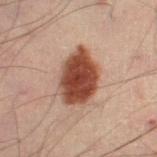Findings:
* follow-up — imaged on a skin check; not biopsied
* patient — male, aged 48–52
* image-analysis metrics — a lesion area of about 18 mm², an outline eccentricity of about 0.75 (0 = round, 1 = elongated), and a shape-asymmetry score of about 0.15 (0 = symmetric); a color-variation rating of about 4/10 and radial color variation of about 1
* lighting — cross-polarized illumination
* diameter — about 6 mm
* body site — the right lower leg
* acquisition — ~15 mm tile from a whole-body skin photo